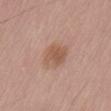biopsy status: catalogued during a skin exam; not biopsied
image source: 15 mm crop, total-body photography
site: the lower back
illumination: white-light illumination
subject: male, approximately 50 years of age